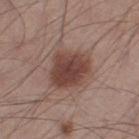The lesion was tiled from a total-body skin photograph and was not biopsied.
A male patient, approximately 45 years of age.
On the right thigh.
This image is a 15 mm lesion crop taken from a total-body photograph.